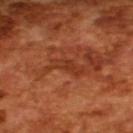Part of a total-body skin-imaging series; this lesion was reviewed on a skin check and was not flagged for biopsy.
Approximately 4.5 mm at its widest.
A region of skin cropped from a whole-body photographic capture, roughly 15 mm wide.
The patient is a male in their mid-60s.